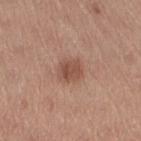follow-up = imaged on a skin check; not biopsied
patient = male, about 60 years old
size = about 3 mm
image source = ~15 mm tile from a whole-body skin photo
illumination = white-light illumination
location = the right thigh